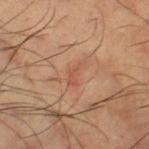{
  "biopsy_status": "not biopsied; imaged during a skin examination",
  "image": {
    "source": "total-body photography crop",
    "field_of_view_mm": 15
  },
  "patient": {
    "sex": "male",
    "age_approx": 65
  },
  "site": "right thigh",
  "lighting": "cross-polarized",
  "automated_metrics": {
    "area_mm2_approx": 3.0,
    "shape_asymmetry": 0.5,
    "nevus_likeness_0_100": 0
  },
  "lesion_size": {
    "long_diameter_mm_approx": 3.0
  }
}